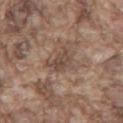Clinical impression: Recorded during total-body skin imaging; not selected for excision or biopsy. Background: Longest diameter approximately 3.5 mm. Located on the front of the torso. A region of skin cropped from a whole-body photographic capture, roughly 15 mm wide. A male patient in their mid-70s. The lesion-visualizer software estimated a shape eccentricity near 0.75. And it measured an automated nevus-likeness rating near 0 out of 100 and a detector confidence of about 85 out of 100 that the crop contains a lesion.